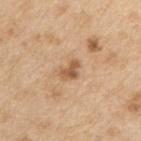{
  "biopsy_status": "not biopsied; imaged during a skin examination",
  "patient": {
    "sex": "male",
    "age_approx": 70
  },
  "lesion_size": {
    "long_diameter_mm_approx": 2.5
  },
  "image": {
    "source": "total-body photography crop",
    "field_of_view_mm": 15
  },
  "site": "right upper arm"
}